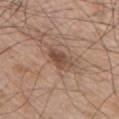The lesion was photographed on a routine skin check and not biopsied; there is no pathology result. The lesion is on the chest. A male patient aged approximately 70. A 15 mm close-up extracted from a 3D total-body photography capture. The tile uses white-light illumination.